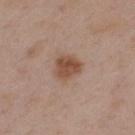No biopsy was performed on this lesion — it was imaged during a full skin examination and was not determined to be concerning. Automated image analysis of the tile measured two-axis asymmetry of about 0.25. It also reported a mean CIELAB color near L≈50 a*≈19 b*≈28 and a normalized border contrast of about 8.5. The analysis additionally found a border-irregularity rating of about 2/10, internal color variation of about 3.5 on a 0–10 scale, and radial color variation of about 1.5. It also reported an automated nevus-likeness rating near 95 out of 100. The patient is a female aged 43–47. The recorded lesion diameter is about 3.5 mm. Cropped from a total-body skin-imaging series; the visible field is about 15 mm. This is a white-light tile. The lesion is located on the upper back.